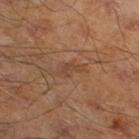Q: Was this lesion biopsied?
A: imaged on a skin check; not biopsied
Q: Where on the body is the lesion?
A: the left lower leg
Q: How large is the lesion?
A: ≈3 mm
Q: What kind of image is this?
A: ~15 mm tile from a whole-body skin photo
Q: What did automated image analysis measure?
A: an area of roughly 3 mm² and a shape eccentricity near 0.85; a lesion color around L≈43 a*≈20 b*≈28 in CIELAB, about 6 CIELAB-L* units darker than the surrounding skin, and a normalized border contrast of about 5; border irregularity of about 5 on a 0–10 scale, internal color variation of about 1 on a 0–10 scale, and a peripheral color-asymmetry measure near 0.5
Q: How was the tile lit?
A: cross-polarized
Q: What are the patient's age and sex?
A: male, approximately 45 years of age